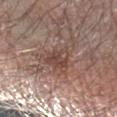No biopsy was performed on this lesion — it was imaged during a full skin examination and was not determined to be concerning. Longest diameter approximately 3 mm. A 15 mm crop from a total-body photograph taken for skin-cancer surveillance. The lesion is on the left forearm. The total-body-photography lesion software estimated a lesion area of about 6.5 mm² and a shape-asymmetry score of about 0.35 (0 = symmetric). It also reported a border-irregularity index near 3.5/10 and radial color variation of about 2. And it measured a classifier nevus-likeness of about 5/100. A male patient, approximately 80 years of age. This is a white-light tile.